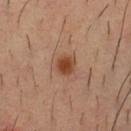Assessment:
The lesion was tiled from a total-body skin photograph and was not biopsied.
Image and clinical context:
This is a cross-polarized tile. The patient is a male approximately 35 years of age. Approximately 2.5 mm at its widest. Cropped from a total-body skin-imaging series; the visible field is about 15 mm. The lesion is on the chest.